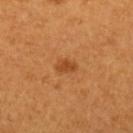Assessment: This lesion was catalogued during total-body skin photography and was not selected for biopsy. Context: The lesion is on the arm. A roughly 15 mm field-of-view crop from a total-body skin photograph. The subject is a female approximately 55 years of age. Imaged with cross-polarized lighting. An algorithmic analysis of the crop reported an area of roughly 3 mm², a shape eccentricity near 0.75, and two-axis asymmetry of about 0.25. And it measured roughly 9 lightness units darker than nearby skin and a normalized border contrast of about 7. The analysis additionally found a border-irregularity index near 2/10, a within-lesion color-variation index near 1/10, and peripheral color asymmetry of about 0.5. The software also gave a nevus-likeness score of about 80/100 and a lesion-detection confidence of about 100/100.